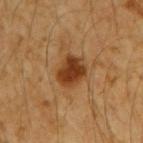notes — total-body-photography surveillance lesion; no biopsy | illumination — cross-polarized illumination | image source — ~15 mm tile from a whole-body skin photo | site — the arm | patient — male, in their 60s.